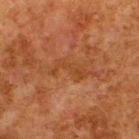Imaged during a routine full-body skin examination; the lesion was not biopsied and no histopathology is available.